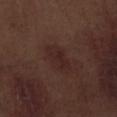The lesion was photographed on a routine skin check and not biopsied; there is no pathology result.